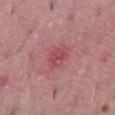Acquisition and patient details: The subject is a male aged 38–42. A 15 mm close-up tile from a total-body photography series done for melanoma screening. The lesion is located on the abdomen.